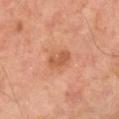Q: Is there a histopathology result?
A: no biopsy performed (imaged during a skin exam)
Q: Who is the patient?
A: male, aged around 50
Q: What is the anatomic site?
A: the left leg
Q: How was this image acquired?
A: ~15 mm tile from a whole-body skin photo